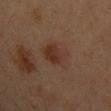{"biopsy_status": "not biopsied; imaged during a skin examination", "automated_metrics": {"vs_skin_darker_L": 6.0, "vs_skin_contrast_norm": 6.0, "nevus_likeness_0_100": 75}, "image": {"source": "total-body photography crop", "field_of_view_mm": 15}, "patient": {"sex": "male", "age_approx": 35}, "lesion_size": {"long_diameter_mm_approx": 4.0}, "site": "chest"}